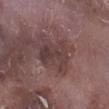biopsy status: catalogued during a skin exam; not biopsied | illumination: white-light | image-analysis metrics: an area of roughly 10 mm², an outline eccentricity of about 0.65 (0 = round, 1 = elongated), and two-axis asymmetry of about 0.35; a border-irregularity index near 5/10, internal color variation of about 4 on a 0–10 scale, and peripheral color asymmetry of about 1.5; lesion-presence confidence of about 100/100 | subject: male, aged around 75 | site: the leg | size: about 4.5 mm | acquisition: ~15 mm crop, total-body skin-cancer survey.